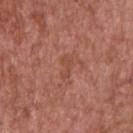<lesion>
  <biopsy_status>not biopsied; imaged during a skin examination</biopsy_status>
</lesion>